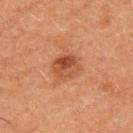Clinical impression:
Part of a total-body skin-imaging series; this lesion was reviewed on a skin check and was not flagged for biopsy.
Image and clinical context:
The lesion is on the left upper arm. A male subject aged around 50. A 15 mm close-up extracted from a 3D total-body photography capture. This is a cross-polarized tile. Approximately 3 mm at its widest.